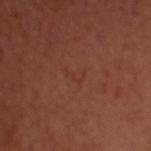Image and clinical context: Cropped from a total-body skin-imaging series; the visible field is about 15 mm. The subject is a male in their mid-60s. Captured under cross-polarized illumination. From the head or neck. The total-body-photography lesion software estimated a footprint of about 2 mm², a shape eccentricity near 0.9, and a shape-asymmetry score of about 0.7 (0 = symmetric). It also reported a border-irregularity rating of about 9.5/10, a within-lesion color-variation index near 0/10, and a peripheral color-asymmetry measure near 0. The analysis additionally found an automated nevus-likeness rating near 0 out of 100.